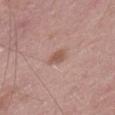Findings:
* notes: total-body-photography surveillance lesion; no biopsy
* lesion diameter: about 2.5 mm
* site: the left thigh
* subject: male, about 50 years old
* illumination: white-light
* image: ~15 mm tile from a whole-body skin photo
* automated metrics: a lesion area of about 3.5 mm², an outline eccentricity of about 0.65 (0 = round, 1 = elongated), and two-axis asymmetry of about 0.3